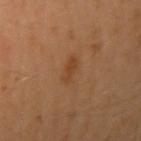The lesion was tiled from a total-body skin photograph and was not biopsied. A male subject approximately 40 years of age. The lesion is located on the right upper arm. A 15 mm close-up tile from a total-body photography series done for melanoma screening. The lesion's longest dimension is about 3 mm.